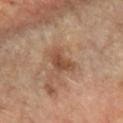No biopsy was performed on this lesion — it was imaged during a full skin examination and was not determined to be concerning. A 15 mm close-up extracted from a 3D total-body photography capture. The patient is a female aged 58–62. The recorded lesion diameter is about 3 mm. This is a cross-polarized tile. On the left forearm.